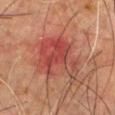Clinical impression:
Recorded during total-body skin imaging; not selected for excision or biopsy.
Clinical summary:
From the chest. A male subject aged around 65. A close-up tile cropped from a whole-body skin photograph, about 15 mm across.